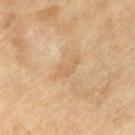The tile uses cross-polarized illumination. The recorded lesion diameter is about 3.5 mm. A roughly 15 mm field-of-view crop from a total-body skin photograph. A male patient, in their mid- to late 60s.